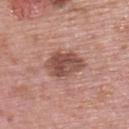| feature | finding |
|---|---|
| lesion size | about 5 mm |
| body site | the upper back |
| image-analysis metrics | a footprint of about 11 mm² and a shape-asymmetry score of about 0.25 (0 = symmetric); a lesion color around L≈50 a*≈23 b*≈25 in CIELAB, a lesion–skin lightness drop of about 13, and a normalized border contrast of about 9; an automated nevus-likeness rating near 60 out of 100 and a lesion-detection confidence of about 100/100 |
| illumination | white-light |
| subject | female, roughly 60 years of age |
| image | total-body-photography crop, ~15 mm field of view |